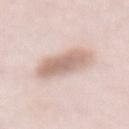  biopsy_status: not biopsied; imaged during a skin examination
  image:
    source: total-body photography crop
    field_of_view_mm: 15
  site: abdomen
  lighting: white-light
  patient:
    sex: female
    age_approx: 65